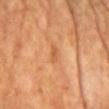Imaged during a routine full-body skin examination; the lesion was not biopsied and no histopathology is available. The lesion is located on the chest. This image is a 15 mm lesion crop taken from a total-body photograph. The lesion's longest dimension is about 2.5 mm. The tile uses cross-polarized illumination. An algorithmic analysis of the crop reported an area of roughly 2 mm² and an outline eccentricity of about 0.95 (0 = round, 1 = elongated). The software also gave a lesion-to-skin contrast of about 6 (normalized; higher = more distinct). The software also gave a border-irregularity rating of about 4/10, a within-lesion color-variation index near 0/10, and peripheral color asymmetry of about 0. A female subject, aged 63–67.